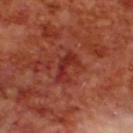| key | value |
|---|---|
| follow-up | catalogued during a skin exam; not biopsied |
| subject | male, about 70 years old |
| image source | total-body-photography crop, ~15 mm field of view |
| tile lighting | cross-polarized illumination |
| location | the upper back |